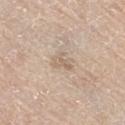Notes:
* follow-up — no biopsy performed (imaged during a skin exam)
* site — the right thigh
* illumination — white-light
* image-analysis metrics — a footprint of about 3.5 mm², an outline eccentricity of about 0.7 (0 = round, 1 = elongated), and two-axis asymmetry of about 0.55; an average lesion color of about L≈63 a*≈13 b*≈29 (CIELAB) and roughly 7 lightness units darker than nearby skin; a border-irregularity rating of about 5.5/10, internal color variation of about 1.5 on a 0–10 scale, and a peripheral color-asymmetry measure near 0.5; an automated nevus-likeness rating near 0 out of 100 and a detector confidence of about 100 out of 100 that the crop contains a lesion
* imaging modality — total-body-photography crop, ~15 mm field of view
* diameter — ≈2.5 mm
* subject — male, approximately 80 years of age